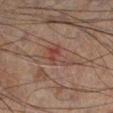notes = imaged on a skin check; not biopsied
patient = male, aged around 60
acquisition = ~15 mm crop, total-body skin-cancer survey
size = ≈5 mm
anatomic site = the left lower leg